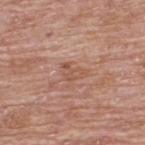Recorded during total-body skin imaging; not selected for excision or biopsy. The subject is a male approximately 65 years of age. The lesion is on the upper back. A 15 mm close-up extracted from a 3D total-body photography capture.